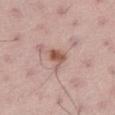workup=catalogued during a skin exam; not biopsied | acquisition=15 mm crop, total-body photography | body site=the left thigh | patient=male, aged around 65.